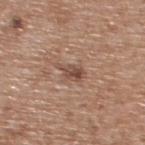biopsy_status: not biopsied; imaged during a skin examination
lesion_size:
  long_diameter_mm_approx: 3.5
site: upper back
patient:
  sex: male
  age_approx: 65
image:
  source: total-body photography crop
  field_of_view_mm: 15